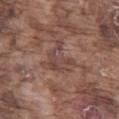workup = imaged on a skin check; not biopsied | image-analysis metrics = an area of roughly 9 mm² and a symmetry-axis asymmetry near 0.5; an average lesion color of about L≈44 a*≈18 b*≈22 (CIELAB) and a lesion–skin lightness drop of about 6; a nevus-likeness score of about 0/100 and lesion-presence confidence of about 75/100 | diameter = ~4.5 mm (longest diameter) | patient = male, approximately 75 years of age | location = the left thigh | image = total-body-photography crop, ~15 mm field of view | tile lighting = white-light illumination.